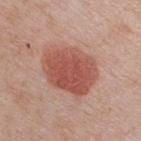Imaged with white-light lighting. A male patient, in their 50s. Longest diameter approximately 6 mm. The lesion is located on the chest. A 15 mm close-up extracted from a 3D total-body photography capture.